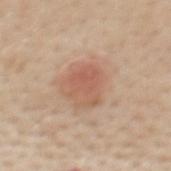Q: Is there a histopathology result?
A: catalogued during a skin exam; not biopsied
Q: How large is the lesion?
A: about 4 mm
Q: Automated lesion metrics?
A: a footprint of about 9.5 mm²; a lesion–skin lightness drop of about 8; internal color variation of about 3 on a 0–10 scale
Q: Lesion location?
A: the mid back
Q: What are the patient's age and sex?
A: female, aged around 50
Q: What is the imaging modality?
A: ~15 mm crop, total-body skin-cancer survey
Q: Illumination type?
A: white-light